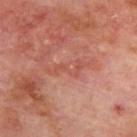Imaged during a routine full-body skin examination; the lesion was not biopsied and no histopathology is available. The tile uses cross-polarized illumination. Cropped from a whole-body photographic skin survey; the tile spans about 15 mm. The total-body-photography lesion software estimated a border-irregularity index near 9/10 and a within-lesion color-variation index near 0/10. The software also gave an automated nevus-likeness rating near 0 out of 100 and a lesion-detection confidence of about 90/100. The subject is a male approximately 70 years of age. On the upper back.